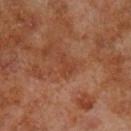notes — imaged on a skin check; not biopsied | acquisition — total-body-photography crop, ~15 mm field of view | illumination — cross-polarized illumination | patient — male, in their 70s | lesion diameter — ~2.5 mm (longest diameter) | anatomic site — the left lower leg.